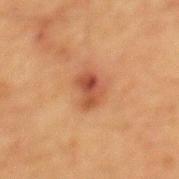notes = no biopsy performed (imaged during a skin exam) | size = about 3.5 mm | imaging modality = total-body-photography crop, ~15 mm field of view | illumination = cross-polarized illumination | body site = the mid back | patient = male, roughly 65 years of age.